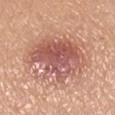Notes:
- notes: catalogued during a skin exam; not biopsied
- image: 15 mm crop, total-body photography
- lighting: white-light
- patient: female, aged 43–47
- body site: the leg
- lesion diameter: ~8 mm (longest diameter)